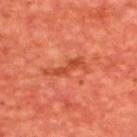Assessment:
Imaged during a routine full-body skin examination; the lesion was not biopsied and no histopathology is available.
Background:
A close-up tile cropped from a whole-body skin photograph, about 15 mm across. From the upper back. The patient is a male about 65 years old.